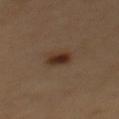Captured during whole-body skin photography for melanoma surveillance; the lesion was not biopsied. A male patient, aged approximately 65. Imaged with cross-polarized lighting. An algorithmic analysis of the crop reported an area of roughly 5 mm² and a shape eccentricity near 0.5. And it measured a mean CIELAB color near L≈30 a*≈16 b*≈25, roughly 10 lightness units darker than nearby skin, and a normalized lesion–skin contrast near 10.5. It also reported a border-irregularity index near 2/10, a within-lesion color-variation index near 3.5/10, and radial color variation of about 1. From the mid back. Longest diameter approximately 2.5 mm. Cropped from a whole-body photographic skin survey; the tile spans about 15 mm.